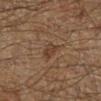<case>
<biopsy_status>not biopsied; imaged during a skin examination</biopsy_status>
<lighting>cross-polarized</lighting>
<lesion_size>
  <long_diameter_mm_approx>2.5</long_diameter_mm_approx>
</lesion_size>
<image>
  <source>total-body photography crop</source>
  <field_of_view_mm>15</field_of_view_mm>
</image>
<site>right lower leg</site>
<patient>
  <sex>male</sex>
  <age_approx>65</age_approx>
</patient>
</case>